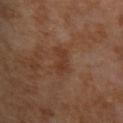Notes:
• notes · no biopsy performed (imaged during a skin exam)
• subject · female, about 55 years old
• site · the back
• tile lighting · cross-polarized
• image source · total-body-photography crop, ~15 mm field of view
• lesion diameter · about 3.5 mm